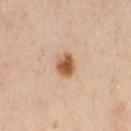Approximately 3 mm at its widest. A 15 mm close-up tile from a total-body photography series done for melanoma screening. The lesion is on the right thigh. Automated tile analysis of the lesion measured an area of roughly 5.5 mm², an eccentricity of roughly 0.55, and two-axis asymmetry of about 0.25. Imaged with cross-polarized lighting. A female patient aged 38–42.